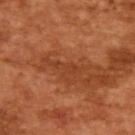notes: catalogued during a skin exam; not biopsied | diameter: about 3.5 mm | illumination: cross-polarized | subject: male, aged approximately 65 | imaging modality: 15 mm crop, total-body photography.